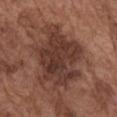The lesion was tiled from a total-body skin photograph and was not biopsied.
On the right upper arm.
Imaged with white-light lighting.
This image is a 15 mm lesion crop taken from a total-body photograph.
Approximately 9.5 mm at its widest.
A male subject, aged around 75.